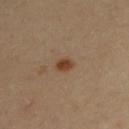Clinical impression:
The lesion was tiled from a total-body skin photograph and was not biopsied.